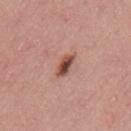A male subject aged 38 to 42. Cropped from a total-body skin-imaging series; the visible field is about 15 mm. The lesion is located on the back.